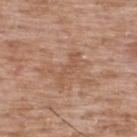The subject is a male aged 48 to 52.
The lesion is on the upper back.
The total-body-photography lesion software estimated a lesion area of about 5 mm², an eccentricity of roughly 0.95, and a symmetry-axis asymmetry near 0.5. The analysis additionally found a lesion color around L≈54 a*≈21 b*≈31 in CIELAB, a lesion–skin lightness drop of about 6, and a normalized lesion–skin contrast near 5. And it measured a border-irregularity index near 7.5/10, a within-lesion color-variation index near 1.5/10, and a peripheral color-asymmetry measure near 0.5.
Imaged with white-light lighting.
The recorded lesion diameter is about 4.5 mm.
Cropped from a whole-body photographic skin survey; the tile spans about 15 mm.